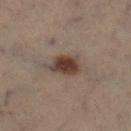Q: Is there a histopathology result?
A: imaged on a skin check; not biopsied
Q: Patient demographics?
A: male, roughly 55 years of age
Q: What is the anatomic site?
A: the right lower leg
Q: Illumination type?
A: cross-polarized illumination
Q: What kind of image is this?
A: total-body-photography crop, ~15 mm field of view
Q: How large is the lesion?
A: about 4 mm